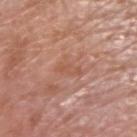Clinical impression:
Recorded during total-body skin imaging; not selected for excision or biopsy.
Background:
Captured under white-light illumination. The lesion's longest dimension is about 3.5 mm. A 15 mm close-up extracted from a 3D total-body photography capture. A male subject approximately 75 years of age. From the left forearm.